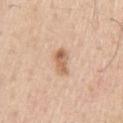No biopsy was performed on this lesion — it was imaged during a full skin examination and was not determined to be concerning. The total-body-photography lesion software estimated a border-irregularity rating of about 2.5/10 and a color-variation rating of about 4.5/10. The analysis additionally found an automated nevus-likeness rating near 80 out of 100 and lesion-presence confidence of about 100/100. Measured at roughly 3.5 mm in maximum diameter. This is a white-light tile. The lesion is located on the right upper arm. The subject is a male aged 63–67. Cropped from a total-body skin-imaging series; the visible field is about 15 mm.